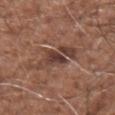follow-up = no biopsy performed (imaged during a skin exam); lesion size = about 5 mm; tile lighting = white-light; image-analysis metrics = a nevus-likeness score of about 0/100 and lesion-presence confidence of about 70/100; imaging modality = ~15 mm tile from a whole-body skin photo; body site = the arm; patient = male, in their mid-70s.